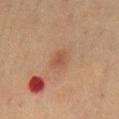Clinical impression: Part of a total-body skin-imaging series; this lesion was reviewed on a skin check and was not flagged for biopsy. Acquisition and patient details: The tile uses cross-polarized illumination. On the back. A region of skin cropped from a whole-body photographic capture, roughly 15 mm wide. The recorded lesion diameter is about 2.5 mm. A male patient roughly 70 years of age.